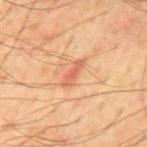follow-up: no biopsy performed (imaged during a skin exam) | body site: the mid back | illumination: cross-polarized illumination | automated metrics: an eccentricity of roughly 0.95 and a shape-asymmetry score of about 0.35 (0 = symmetric); a mean CIELAB color near L≈49 a*≈23 b*≈31, a lesion–skin lightness drop of about 8, and a normalized border contrast of about 6 | lesion diameter: ~3.5 mm (longest diameter) | patient: male, aged 63 to 67 | image source: ~15 mm tile from a whole-body skin photo.